The lesion is located on the right forearm, the subject is a female aged 38–42, a 15 mm close-up tile from a total-body photography series done for melanoma screening: 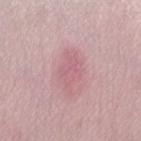This is a white-light tile.
Approximately 4.5 mm at its widest.
An algorithmic analysis of the crop reported an area of roughly 8 mm² and an eccentricity of roughly 0.8. The software also gave a lesion color around L≈61 a*≈25 b*≈17 in CIELAB, a lesion–skin lightness drop of about 7, and a lesion-to-skin contrast of about 4.5 (normalized; higher = more distinct). The analysis additionally found a border-irregularity rating of about 3.5/10, a color-variation rating of about 2.5/10, and radial color variation of about 1. It also reported a classifier nevus-likeness of about 5/100 and a detector confidence of about 95 out of 100 that the crop contains a lesion.
The biopsy diagnosis was a dermatofibroma.